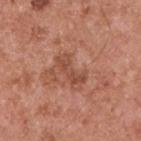Imaged during a routine full-body skin examination; the lesion was not biopsied and no histopathology is available.
About 4 mm across.
On the upper back.
The lesion-visualizer software estimated border irregularity of about 7 on a 0–10 scale and radial color variation of about 1.
A close-up tile cropped from a whole-body skin photograph, about 15 mm across.
A male subject in their mid-50s.